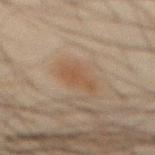Captured during whole-body skin photography for melanoma surveillance; the lesion was not biopsied.
Captured under cross-polarized illumination.
About 3.5 mm across.
Automated tile analysis of the lesion measured a symmetry-axis asymmetry near 0.35. And it measured a mean CIELAB color near L≈41 a*≈15 b*≈27. And it measured a color-variation rating of about 1.5/10 and a peripheral color-asymmetry measure near 0.5. It also reported a classifier nevus-likeness of about 95/100.
Located on the abdomen.
A roughly 15 mm field-of-view crop from a total-body skin photograph.
The subject is a male aged 43–47.